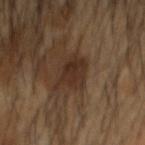Clinical impression:
Imaged during a routine full-body skin examination; the lesion was not biopsied and no histopathology is available.
Context:
A 15 mm close-up tile from a total-body photography series done for melanoma screening. On the head or neck. A male patient aged approximately 55. Captured under cross-polarized illumination. Approximately 4 mm at its widest. Automated image analysis of the tile measured a lesion area of about 8 mm², an outline eccentricity of about 0.55 (0 = round, 1 = elongated), and a shape-asymmetry score of about 0.35 (0 = symmetric). The software also gave a nevus-likeness score of about 5/100.